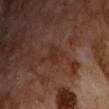The recorded lesion diameter is about 2.5 mm. On the chest. A close-up tile cropped from a whole-body skin photograph, about 15 mm across. A male patient approximately 70 years of age. Imaged with cross-polarized lighting.